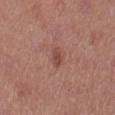Impression: The lesion was photographed on a routine skin check and not biopsied; there is no pathology result. Acquisition and patient details: The total-body-photography lesion software estimated a classifier nevus-likeness of about 0/100 and lesion-presence confidence of about 100/100. Longest diameter approximately 2.5 mm. Imaged with white-light lighting. A 15 mm close-up tile from a total-body photography series done for melanoma screening. Located on the leg. A female patient approximately 40 years of age.